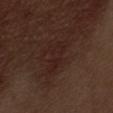This lesion was catalogued during total-body skin photography and was not selected for biopsy. Captured under white-light illumination. Automated image analysis of the tile measured a border-irregularity index near 5.5/10, a within-lesion color-variation index near 1.5/10, and a peripheral color-asymmetry measure near 0.5. Located on the abdomen. A 15 mm close-up tile from a total-body photography series done for melanoma screening. Approximately 4.5 mm at its widest. A male patient, aged approximately 70.